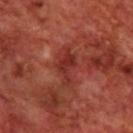- biopsy status · total-body-photography surveillance lesion; no biopsy
- automated metrics · a footprint of about 7 mm² and two-axis asymmetry of about 0.45; a lesion color around L≈33 a*≈31 b*≈28 in CIELAB and a normalized border contrast of about 7
- subject · male, roughly 70 years of age
- lighting · cross-polarized illumination
- site · the back
- image · 15 mm crop, total-body photography
- lesion size · ~4.5 mm (longest diameter)